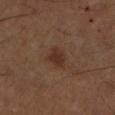Clinical impression:
This lesion was catalogued during total-body skin photography and was not selected for biopsy.
Image and clinical context:
Approximately 2.5 mm at its widest. The lesion is on the left lower leg. A male patient, roughly 50 years of age. A 15 mm close-up tile from a total-body photography series done for melanoma screening.